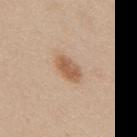Recorded during total-body skin imaging; not selected for excision or biopsy.
Measured at roughly 4 mm in maximum diameter.
From the mid back.
Automated image analysis of the tile measured a mean CIELAB color near L≈58 a*≈19 b*≈33 and a lesion-to-skin contrast of about 8 (normalized; higher = more distinct). The analysis additionally found a border-irregularity index near 1.5/10, a color-variation rating of about 3/10, and radial color variation of about 1.
Captured under white-light illumination.
A roughly 15 mm field-of-view crop from a total-body skin photograph.
A female patient aged approximately 40.